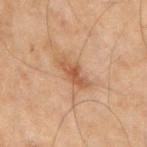This lesion was catalogued during total-body skin photography and was not selected for biopsy.
Located on the right upper arm.
Approximately 4 mm at its widest.
The subject is a male approximately 70 years of age.
Cropped from a whole-body photographic skin survey; the tile spans about 15 mm.